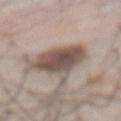Part of a total-body skin-imaging series; this lesion was reviewed on a skin check and was not flagged for biopsy. From the front of the torso. A 15 mm close-up tile from a total-body photography series done for melanoma screening. Approximately 5 mm at its widest. Captured under white-light illumination. A male subject aged 63 to 67.